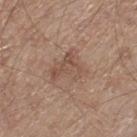The lesion-visualizer software estimated a footprint of about 9.5 mm², an eccentricity of roughly 0.65, and a shape-asymmetry score of about 0.55 (0 = symmetric). It also reported roughly 8 lightness units darker than nearby skin. It also reported lesion-presence confidence of about 100/100.
A region of skin cropped from a whole-body photographic capture, roughly 15 mm wide.
Captured under white-light illumination.
Located on the left thigh.
A male subject aged approximately 65.
Measured at roughly 4.5 mm in maximum diameter.